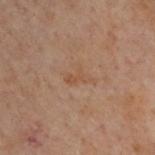  biopsy_status: not biopsied; imaged during a skin examination
  site: back
  image:
    source: total-body photography crop
    field_of_view_mm: 15
  lighting: cross-polarized
  patient:
    sex: male
    age_approx: 50